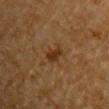Clinical impression:
Captured during whole-body skin photography for melanoma surveillance; the lesion was not biopsied.
Image and clinical context:
A male patient aged approximately 85 or older. A close-up tile cropped from a whole-body skin photograph, about 15 mm across. Measured at roughly 3 mm in maximum diameter. The tile uses cross-polarized illumination. Located on the chest.